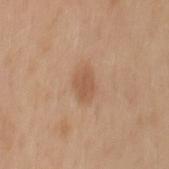Case summary:
- follow-up — imaged on a skin check; not biopsied
- diameter — ≈3.5 mm
- lighting — white-light illumination
- subject — male, approximately 30 years of age
- acquisition — ~15 mm tile from a whole-body skin photo
- location — the mid back